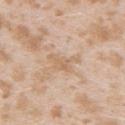A region of skin cropped from a whole-body photographic capture, roughly 15 mm wide.
Automated tile analysis of the lesion measured a footprint of about 5 mm² and an outline eccentricity of about 0.85 (0 = round, 1 = elongated). It also reported an average lesion color of about L≈64 a*≈17 b*≈33 (CIELAB) and a lesion–skin lightness drop of about 6. It also reported a border-irregularity index near 6.5/10, a within-lesion color-variation index near 1.5/10, and peripheral color asymmetry of about 0.5.
A female subject, aged 23–27.
Located on the right upper arm.